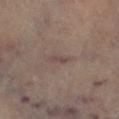  biopsy_status: not biopsied; imaged during a skin examination
  patient:
    sex: male
    age_approx: 65
  image:
    source: total-body photography crop
    field_of_view_mm: 15
  site: right lower leg
  lighting: cross-polarized